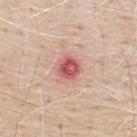This lesion was catalogued during total-body skin photography and was not selected for biopsy. A close-up tile cropped from a whole-body skin photograph, about 15 mm across. The lesion is located on the upper back. A male subject in their mid-60s.